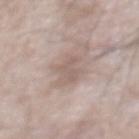Q: Was this lesion biopsied?
A: catalogued during a skin exam; not biopsied
Q: Lesion location?
A: the chest
Q: How large is the lesion?
A: ≈3 mm
Q: Patient demographics?
A: male, approximately 50 years of age
Q: What kind of image is this?
A: ~15 mm tile from a whole-body skin photo